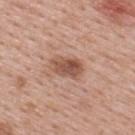notes=catalogued during a skin exam; not biopsied
site=the upper back
image=15 mm crop, total-body photography
tile lighting=white-light illumination
TBP lesion metrics=a nevus-likeness score of about 85/100 and lesion-presence confidence of about 100/100
patient=male, aged around 40